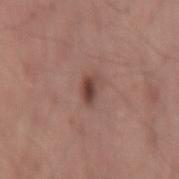Part of a total-body skin-imaging series; this lesion was reviewed on a skin check and was not flagged for biopsy.
A close-up tile cropped from a whole-body skin photograph, about 15 mm across.
A male subject, aged 53 to 57.
From the right thigh.
The lesion-visualizer software estimated a lesion area of about 3 mm², a shape eccentricity near 0.85, and a shape-asymmetry score of about 0.2 (0 = symmetric). The software also gave an average lesion color of about L≈42 a*≈20 b*≈23 (CIELAB), a lesion–skin lightness drop of about 12, and a normalized lesion–skin contrast near 9.5. It also reported a color-variation rating of about 3.5/10. The software also gave an automated nevus-likeness rating near 100 out of 100 and lesion-presence confidence of about 100/100.
Captured under white-light illumination.